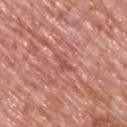Q: Was a biopsy performed?
A: no biopsy performed (imaged during a skin exam)
Q: What is the lesion's diameter?
A: ~3 mm (longest diameter)
Q: Patient demographics?
A: male, aged 73–77
Q: Lesion location?
A: the upper back
Q: How was the tile lit?
A: white-light illumination
Q: How was this image acquired?
A: 15 mm crop, total-body photography